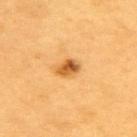follow-up — no biopsy performed (imaged during a skin exam) | acquisition — total-body-photography crop, ~15 mm field of view | site — the upper back | subject — male, aged approximately 60.